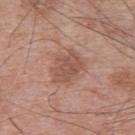{
  "biopsy_status": "not biopsied; imaged during a skin examination"
}